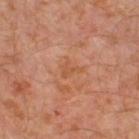| feature | finding |
|---|---|
| workup | no biopsy performed (imaged during a skin exam) |
| diameter | ≈2.5 mm |
| image | ~15 mm crop, total-body skin-cancer survey |
| subject | male, about 30 years old |
| lighting | cross-polarized illumination |
| body site | the left leg |
| automated lesion analysis | an eccentricity of roughly 0.7; a lesion color around L≈52 a*≈25 b*≈35 in CIELAB, about 6 CIELAB-L* units darker than the surrounding skin, and a lesion-to-skin contrast of about 5 (normalized; higher = more distinct); lesion-presence confidence of about 100/100 |